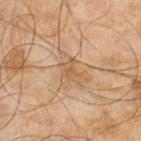Assessment:
Recorded during total-body skin imaging; not selected for excision or biopsy.
Image and clinical context:
The tile uses cross-polarized illumination. The recorded lesion diameter is about 3 mm. An algorithmic analysis of the crop reported a lesion area of about 4 mm² and an outline eccentricity of about 0.75 (0 = round, 1 = elongated). It also reported a lesion color around L≈46 a*≈15 b*≈29 in CIELAB, roughly 6 lightness units darker than nearby skin, and a normalized lesion–skin contrast near 5.5. The analysis additionally found border irregularity of about 4 on a 0–10 scale and internal color variation of about 1.5 on a 0–10 scale. The software also gave an automated nevus-likeness rating near 0 out of 100 and lesion-presence confidence of about 95/100. Cropped from a total-body skin-imaging series; the visible field is about 15 mm. A male patient, roughly 45 years of age. On the right lower leg.